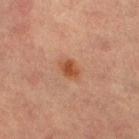Part of a total-body skin-imaging series; this lesion was reviewed on a skin check and was not flagged for biopsy. Imaged with cross-polarized lighting. The lesion is on the left lower leg. A female patient about 60 years old. A region of skin cropped from a whole-body photographic capture, roughly 15 mm wide.